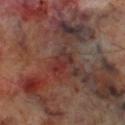Notes:
• biopsy status · no biopsy performed (imaged during a skin exam)
• location · the right lower leg
• acquisition · ~15 mm crop, total-body skin-cancer survey
• subject · male, aged 68 to 72